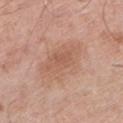biopsy_status: not biopsied; imaged during a skin examination
patient:
  sex: male
  age_approx: 80
automated_metrics:
  area_mm2_approx: 19.0
  eccentricity: 0.8
  shape_asymmetry: 0.25
  cielab_L: 59
  cielab_a: 21
  cielab_b: 30
  vs_skin_darker_L: 7.0
  vs_skin_contrast_norm: 5.0
  nevus_likeness_0_100: 0
  lesion_detection_confidence_0_100: 100
site: left lower leg
image:
  source: total-body photography crop
  field_of_view_mm: 15
lesion_size:
  long_diameter_mm_approx: 6.5
lighting: white-light